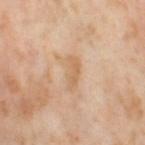Assessment: Captured during whole-body skin photography for melanoma surveillance; the lesion was not biopsied. Acquisition and patient details: A 15 mm close-up extracted from a 3D total-body photography capture. Imaged with cross-polarized lighting. The lesion-visualizer software estimated a footprint of about 5 mm², an outline eccentricity of about 0.9 (0 = round, 1 = elongated), and a symmetry-axis asymmetry near 0.35. The software also gave an average lesion color of about L≈65 a*≈19 b*≈36 (CIELAB), roughly 7 lightness units darker than nearby skin, and a normalized lesion–skin contrast near 5.5. From the right thigh. A female patient aged 53 to 57.